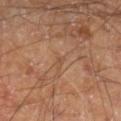Part of a total-body skin-imaging series; this lesion was reviewed on a skin check and was not flagged for biopsy.
A male patient, about 60 years old.
A close-up tile cropped from a whole-body skin photograph, about 15 mm across.
On the right lower leg.